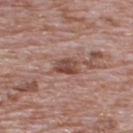Q: Is there a histopathology result?
A: total-body-photography surveillance lesion; no biopsy
Q: What is the lesion's diameter?
A: ≈2.5 mm
Q: Automated lesion metrics?
A: border irregularity of about 2 on a 0–10 scale, a within-lesion color-variation index near 4/10, and peripheral color asymmetry of about 1.5
Q: How was the tile lit?
A: white-light
Q: What kind of image is this?
A: ~15 mm crop, total-body skin-cancer survey
Q: Who is the patient?
A: male, aged around 70
Q: Where on the body is the lesion?
A: the upper back